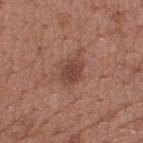Part of a total-body skin-imaging series; this lesion was reviewed on a skin check and was not flagged for biopsy.
The lesion is located on the upper back.
A 15 mm crop from a total-body photograph taken for skin-cancer surveillance.
The lesion's longest dimension is about 3 mm.
A male subject, about 65 years old.
The lesion-visualizer software estimated an area of roughly 6.5 mm² and an outline eccentricity of about 0.55 (0 = round, 1 = elongated). It also reported a mean CIELAB color near L≈44 a*≈21 b*≈25 and a normalized lesion–skin contrast near 7.5.